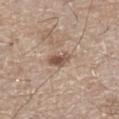biopsy status = imaged on a skin check; not biopsied | image = ~15 mm crop, total-body skin-cancer survey | body site = the left lower leg | subject = male, aged 63 to 67 | size = ≈2.5 mm | TBP lesion metrics = an average lesion color of about L≈52 a*≈17 b*≈27 (CIELAB), roughly 12 lightness units darker than nearby skin, and a normalized border contrast of about 8.5; a color-variation rating of about 3/10; a classifier nevus-likeness of about 45/100 and lesion-presence confidence of about 100/100.